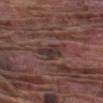No biopsy was performed on this lesion — it was imaged during a full skin examination and was not determined to be concerning.
Located on the left forearm.
A 15 mm close-up tile from a total-body photography series done for melanoma screening.
A male patient, aged approximately 75.